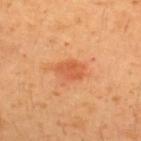biopsy status: total-body-photography surveillance lesion; no biopsy
location: the upper back
imaging modality: total-body-photography crop, ~15 mm field of view
automated lesion analysis: a lesion color around L≈57 a*≈31 b*≈41 in CIELAB, roughly 9 lightness units darker than nearby skin, and a normalized border contrast of about 6; an automated nevus-likeness rating near 65 out of 100 and lesion-presence confidence of about 100/100
lighting: cross-polarized illumination
subject: male, about 30 years old